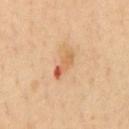A 15 mm crop from a total-body photograph taken for skin-cancer surveillance.
On the chest.
A male subject roughly 50 years of age.